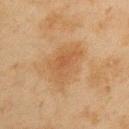Imaged during a routine full-body skin examination; the lesion was not biopsied and no histopathology is available.
From the left upper arm.
Cropped from a total-body skin-imaging series; the visible field is about 15 mm.
Imaged with cross-polarized lighting.
A male subject aged around 45.